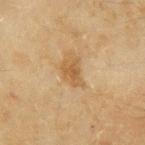{"biopsy_status": "not biopsied; imaged during a skin examination", "lighting": "cross-polarized", "image": {"source": "total-body photography crop", "field_of_view_mm": 15}, "lesion_size": {"long_diameter_mm_approx": 3.0}, "patient": {"sex": "female", "age_approx": 55}, "site": "left upper arm"}